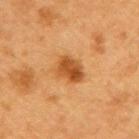follow-up = total-body-photography surveillance lesion; no biopsy